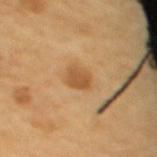follow-up: total-body-photography surveillance lesion; no biopsy | image source: 15 mm crop, total-body photography | anatomic site: the back | patient: female, in their mid- to late 60s | tile lighting: cross-polarized illumination | size: ≈2.5 mm | TBP lesion metrics: a lesion color around L≈52 a*≈21 b*≈39 in CIELAB, roughly 10 lightness units darker than nearby skin, and a lesion-to-skin contrast of about 7 (normalized; higher = more distinct).